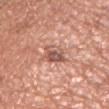– notes: catalogued during a skin exam; not biopsied
– subject: male, aged around 65
– automated metrics: an area of roughly 6.5 mm², an outline eccentricity of about 0.85 (0 = round, 1 = elongated), and a symmetry-axis asymmetry near 0.25; about 12 CIELAB-L* units darker than the surrounding skin and a normalized border contrast of about 8; a color-variation rating of about 6.5/10 and peripheral color asymmetry of about 2.5; a classifier nevus-likeness of about 0/100 and a detector confidence of about 95 out of 100 that the crop contains a lesion
– location: the right upper arm
– image source: ~15 mm tile from a whole-body skin photo
– lighting: white-light